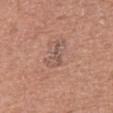Clinical impression:
This lesion was catalogued during total-body skin photography and was not selected for biopsy.
Image and clinical context:
The tile uses white-light illumination. Located on the left thigh. The lesion's longest dimension is about 3.5 mm. A female patient, roughly 50 years of age. A 15 mm crop from a total-body photograph taken for skin-cancer surveillance. The total-body-photography lesion software estimated an average lesion color of about L≈53 a*≈19 b*≈25 (CIELAB), a lesion–skin lightness drop of about 7, and a normalized lesion–skin contrast near 5.5. It also reported a border-irregularity rating of about 8.5/10, internal color variation of about 2.5 on a 0–10 scale, and radial color variation of about 1.